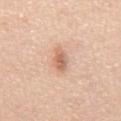workup: no biopsy performed (imaged during a skin exam) | imaging modality: 15 mm crop, total-body photography | body site: the abdomen | patient: male, aged 48–52 | image-analysis metrics: an area of roughly 4.5 mm², an outline eccentricity of about 0.9 (0 = round, 1 = elongated), and a shape-asymmetry score of about 0.25 (0 = symmetric); an average lesion color of about L≈64 a*≈21 b*≈32 (CIELAB) and about 12 CIELAB-L* units darker than the surrounding skin; a within-lesion color-variation index near 3/10 and peripheral color asymmetry of about 1; a classifier nevus-likeness of about 35/100 and lesion-presence confidence of about 100/100 | tile lighting: white-light illumination | lesion size: ≈3.5 mm.